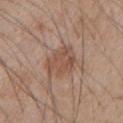biopsy status = total-body-photography surveillance lesion; no biopsy | subject = male, roughly 65 years of age | lighting = white-light | imaging modality = ~15 mm tile from a whole-body skin photo | lesion size = ≈4 mm | site = the chest.